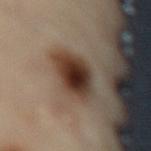Assessment: The lesion was photographed on a routine skin check and not biopsied; there is no pathology result. Acquisition and patient details: A female patient aged 58–62. The lesion-visualizer software estimated a mean CIELAB color near L≈35 a*≈15 b*≈24, roughly 15 lightness units darker than nearby skin, and a lesion-to-skin contrast of about 13 (normalized; higher = more distinct). The software also gave border irregularity of about 2 on a 0–10 scale. A close-up tile cropped from a whole-body skin photograph, about 15 mm across. The tile uses cross-polarized illumination. Located on the lower back.